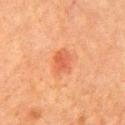The lesion was tiled from a total-body skin photograph and was not biopsied.
The recorded lesion diameter is about 3 mm.
A 15 mm close-up extracted from a 3D total-body photography capture.
An algorithmic analysis of the crop reported an area of roughly 6 mm², an outline eccentricity of about 0.7 (0 = round, 1 = elongated), and two-axis asymmetry of about 0.2. It also reported roughly 7 lightness units darker than nearby skin and a normalized border contrast of about 6.
A male patient, aged around 80.
Imaged with cross-polarized lighting.
From the chest.